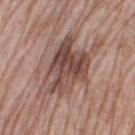biopsy status: imaged on a skin check; not biopsied | anatomic site: the right thigh | patient: female, about 75 years old | automated lesion analysis: a footprint of about 21 mm², an outline eccentricity of about 0.75 (0 = round, 1 = elongated), and two-axis asymmetry of about 0.35; a border-irregularity index near 5.5/10, internal color variation of about 7 on a 0–10 scale, and a peripheral color-asymmetry measure near 2.5 | imaging modality: 15 mm crop, total-body photography | lighting: white-light.